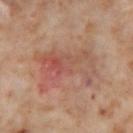Q: Is there a histopathology result?
A: imaged on a skin check; not biopsied
Q: What kind of image is this?
A: 15 mm crop, total-body photography
Q: Automated lesion metrics?
A: an area of roughly 25 mm² and an outline eccentricity of about 0.8 (0 = round, 1 = elongated); border irregularity of about 5.5 on a 0–10 scale and internal color variation of about 9 on a 0–10 scale; a nevus-likeness score of about 10/100 and a lesion-detection confidence of about 100/100
Q: Lesion location?
A: the right thigh
Q: Patient demographics?
A: female, about 55 years old
Q: Illumination type?
A: cross-polarized illumination